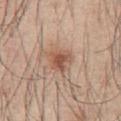Clinical impression: The lesion was tiled from a total-body skin photograph and was not biopsied. Clinical summary: From the chest. This image is a 15 mm lesion crop taken from a total-body photograph. The subject is a male in their mid- to late 40s. The recorded lesion diameter is about 3 mm. Automated image analysis of the tile measured a footprint of about 5.5 mm² and a shape eccentricity near 0.45. The analysis additionally found a border-irregularity rating of about 3/10, a within-lesion color-variation index near 3.5/10, and a peripheral color-asymmetry measure near 1.